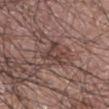Part of a total-body skin-imaging series; this lesion was reviewed on a skin check and was not flagged for biopsy. Cropped from a whole-body photographic skin survey; the tile spans about 15 mm. The lesion's longest dimension is about 3.5 mm. From the left arm. The tile uses white-light illumination. The subject is a male aged 68 to 72.